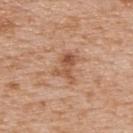Case summary:
- notes: catalogued during a skin exam; not biopsied
- tile lighting: white-light
- size: ≈3.5 mm
- location: the upper back
- patient: male, aged around 65
- imaging modality: total-body-photography crop, ~15 mm field of view